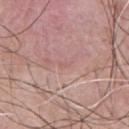follow-up = imaged on a skin check; not biopsied | size = ~1.5 mm (longest diameter) | tile lighting = white-light | TBP lesion metrics = a footprint of about 1 mm², a shape eccentricity near 0.85, and a symmetry-axis asymmetry near 0.3; a border-irregularity index near 2.5/10 and a color-variation rating of about 0/10; a classifier nevus-likeness of about 0/100 and a detector confidence of about 80 out of 100 that the crop contains a lesion | image source = ~15 mm crop, total-body skin-cancer survey | patient = male, about 45 years old | location = the back.